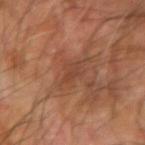Approximately 2.5 mm at its widest.
The subject is a male aged approximately 70.
A 15 mm crop from a total-body photograph taken for skin-cancer surveillance.
On the left forearm.
An algorithmic analysis of the crop reported an area of roughly 2 mm² and a symmetry-axis asymmetry near 0.4.